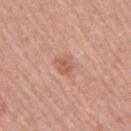| key | value |
|---|---|
| workup | imaged on a skin check; not biopsied |
| location | the arm |
| lighting | white-light illumination |
| patient | male, roughly 60 years of age |
| size | ~2.5 mm (longest diameter) |
| acquisition | ~15 mm tile from a whole-body skin photo |
| TBP lesion metrics | a footprint of about 4.5 mm², an eccentricity of roughly 0.5, and a shape-asymmetry score of about 0.3 (0 = symmetric); a border-irregularity index near 2.5/10, internal color variation of about 3.5 on a 0–10 scale, and peripheral color asymmetry of about 1; a classifier nevus-likeness of about 35/100 and a lesion-detection confidence of about 100/100 |